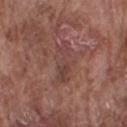biopsy status — total-body-photography surveillance lesion; no biopsy | patient — male, approximately 75 years of age | tile lighting — white-light illumination | lesion size — ≈5 mm | acquisition — ~15 mm crop, total-body skin-cancer survey | anatomic site — the right upper arm.